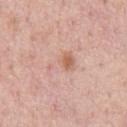A region of skin cropped from a whole-body photographic capture, roughly 15 mm wide. This is a white-light tile. The subject is a male aged 38 to 42. The lesion is on the front of the torso.